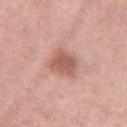Imaged during a routine full-body skin examination; the lesion was not biopsied and no histopathology is available.
A female subject about 40 years old.
The recorded lesion diameter is about 4 mm.
A 15 mm crop from a total-body photograph taken for skin-cancer surveillance.
Automated image analysis of the tile measured a footprint of about 9.5 mm², an outline eccentricity of about 0.55 (0 = round, 1 = elongated), and a shape-asymmetry score of about 0.25 (0 = symmetric). It also reported a mean CIELAB color near L≈58 a*≈23 b*≈26, about 11 CIELAB-L* units darker than the surrounding skin, and a normalized lesion–skin contrast near 7.5.
From the right thigh.
Captured under white-light illumination.